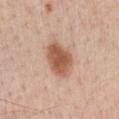notes = imaged on a skin check; not biopsied | illumination = white-light illumination | acquisition = 15 mm crop, total-body photography | TBP lesion metrics = an area of roughly 11 mm², an eccentricity of roughly 0.75, and two-axis asymmetry of about 0.15 | subject = male, aged around 40 | body site = the front of the torso.